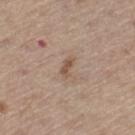An algorithmic analysis of the crop reported a border-irregularity rating of about 3/10, internal color variation of about 0 on a 0–10 scale, and radial color variation of about 0. It also reported a classifier nevus-likeness of about 0/100 and a detector confidence of about 100 out of 100 that the crop contains a lesion. A roughly 15 mm field-of-view crop from a total-body skin photograph. This is a white-light tile. A male patient, aged 68–72. On the left thigh. The lesion's longest dimension is about 2.5 mm.